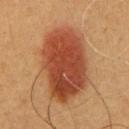No biopsy was performed on this lesion — it was imaged during a full skin examination and was not determined to be concerning.
On the chest.
The total-body-photography lesion software estimated an area of roughly 40 mm², an outline eccentricity of about 0.8 (0 = round, 1 = elongated), and a shape-asymmetry score of about 0.15 (0 = symmetric). It also reported a mean CIELAB color near L≈42 a*≈26 b*≈33, a lesion–skin lightness drop of about 14, and a normalized lesion–skin contrast near 10.5. The analysis additionally found a border-irregularity rating of about 2/10, internal color variation of about 5.5 on a 0–10 scale, and radial color variation of about 1.5. And it measured lesion-presence confidence of about 100/100.
A male subject, aged around 60.
About 9.5 mm across.
The tile uses cross-polarized illumination.
A lesion tile, about 15 mm wide, cut from a 3D total-body photograph.